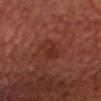Impression:
Recorded during total-body skin imaging; not selected for excision or biopsy.
Background:
Measured at roughly 3 mm in maximum diameter. From the head or neck. The tile uses cross-polarized illumination. A lesion tile, about 15 mm wide, cut from a 3D total-body photograph. The patient is a male in their mid-60s.